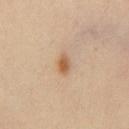Clinical impression: This lesion was catalogued during total-body skin photography and was not selected for biopsy. Image and clinical context: A 15 mm close-up tile from a total-body photography series done for melanoma screening. The recorded lesion diameter is about 3 mm. On the chest. A male patient in their 50s. The total-body-photography lesion software estimated a lesion area of about 3.5 mm², an outline eccentricity of about 0.85 (0 = round, 1 = elongated), and a symmetry-axis asymmetry near 0.15. It also reported a lesion color around L≈57 a*≈19 b*≈35 in CIELAB, about 11 CIELAB-L* units darker than the surrounding skin, and a normalized border contrast of about 8. The analysis additionally found a border-irregularity rating of about 1.5/10, a within-lesion color-variation index near 2.5/10, and a peripheral color-asymmetry measure near 1. This is a cross-polarized tile.